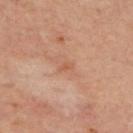{"biopsy_status": "not biopsied; imaged during a skin examination", "automated_metrics": {"border_irregularity_0_10": 3.0, "color_variation_0_10": 0.0, "peripheral_color_asymmetry": 0.0, "nevus_likeness_0_100": 0, "lesion_detection_confidence_0_100": 100}, "image": {"source": "total-body photography crop", "field_of_view_mm": 15}, "site": "upper back", "lighting": "cross-polarized", "patient": {"sex": "female", "age_approx": 45}, "lesion_size": {"long_diameter_mm_approx": 2.0}}